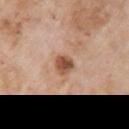Assessment: Part of a total-body skin-imaging series; this lesion was reviewed on a skin check and was not flagged for biopsy.